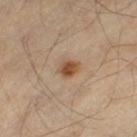<lesion>
<biopsy_status>not biopsied; imaged during a skin examination</biopsy_status>
<lighting>cross-polarized</lighting>
<image>
  <source>total-body photography crop</source>
  <field_of_view_mm>15</field_of_view_mm>
</image>
<automated_metrics>
  <cielab_L>49</cielab_L>
  <cielab_a>20</cielab_a>
  <cielab_b>33</cielab_b>
  <vs_skin_darker_L>12.0</vs_skin_darker_L>
  <vs_skin_contrast_norm>9.5</vs_skin_contrast_norm>
  <nevus_likeness_0_100>90</nevus_likeness_0_100>
  <lesion_detection_confidence_0_100>100</lesion_detection_confidence_0_100>
</automated_metrics>
<site>leg</site>
<lesion_size>
  <long_diameter_mm_approx>2.5</long_diameter_mm_approx>
</lesion_size>
<patient>
  <sex>male</sex>
  <age_approx>55</age_approx>
</patient>
</lesion>